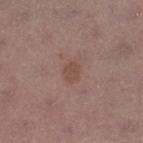Assessment:
No biopsy was performed on this lesion — it was imaged during a full skin examination and was not determined to be concerning.